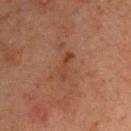Impression: Recorded during total-body skin imaging; not selected for excision or biopsy. Context: Located on the left upper arm. A close-up tile cropped from a whole-body skin photograph, about 15 mm across. A male subject aged 58–62.